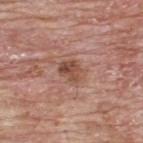Part of a total-body skin-imaging series; this lesion was reviewed on a skin check and was not flagged for biopsy.